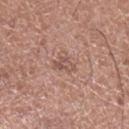notes = no biopsy performed (imaged during a skin exam)
site = the leg
image source = ~15 mm crop, total-body skin-cancer survey
subject = female, about 50 years old